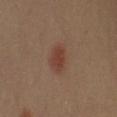- workup · imaged on a skin check; not biopsied
- subject · male, approximately 40 years of age
- anatomic site · the arm
- lesion diameter · about 3 mm
- image source · 15 mm crop, total-body photography